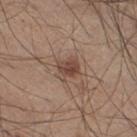Assessment: Imaged during a routine full-body skin examination; the lesion was not biopsied and no histopathology is available. Image and clinical context: The subject is a male in their mid- to late 40s. A close-up tile cropped from a whole-body skin photograph, about 15 mm across. Located on the right thigh. Automated tile analysis of the lesion measured an average lesion color of about L≈45 a*≈18 b*≈25 (CIELAB), a lesion–skin lightness drop of about 10, and a normalized border contrast of about 7.5. The analysis additionally found a border-irregularity index near 2.5/10, a within-lesion color-variation index near 3.5/10, and peripheral color asymmetry of about 1.5. The software also gave a nevus-likeness score of about 85/100 and lesion-presence confidence of about 100/100. Measured at roughly 3 mm in maximum diameter. The tile uses white-light illumination.